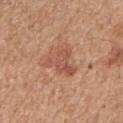<case>
  <biopsy_status>not biopsied; imaged during a skin examination</biopsy_status>
  <image>
    <source>total-body photography crop</source>
    <field_of_view_mm>15</field_of_view_mm>
  </image>
  <site>arm</site>
  <patient>
    <sex>female</sex>
    <age_approx>50</age_approx>
  </patient>
</case>